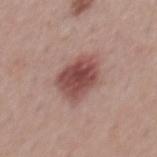Assessment: The lesion was tiled from a total-body skin photograph and was not biopsied. Clinical summary: An algorithmic analysis of the crop reported a lesion area of about 14 mm² and a symmetry-axis asymmetry near 0.2. And it measured border irregularity of about 2 on a 0–10 scale, a color-variation rating of about 4/10, and radial color variation of about 1. The analysis additionally found lesion-presence confidence of about 100/100. The patient is a male in their mid-50s. The tile uses white-light illumination. A close-up tile cropped from a whole-body skin photograph, about 15 mm across. The lesion is located on the back.